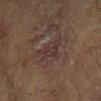This lesion was catalogued during total-body skin photography and was not selected for biopsy.